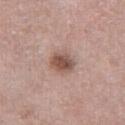Assessment:
Recorded during total-body skin imaging; not selected for excision or biopsy.
Acquisition and patient details:
The recorded lesion diameter is about 3 mm. The tile uses white-light illumination. A close-up tile cropped from a whole-body skin photograph, about 15 mm across. The subject is a female approximately 60 years of age. The lesion is located on the right thigh.